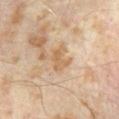{"biopsy_status": "not biopsied; imaged during a skin examination", "lesion_size": {"long_diameter_mm_approx": 3.0}, "automated_metrics": {"border_irregularity_0_10": 4.0, "color_variation_0_10": 2.5, "peripheral_color_asymmetry": 1.0}, "image": {"source": "total-body photography crop", "field_of_view_mm": 15}, "lighting": "cross-polarized", "patient": {"sex": "male", "age_approx": 65}}